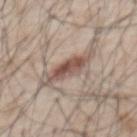| key | value |
|---|---|
| biopsy status | imaged on a skin check; not biopsied |
| patient | male, roughly 65 years of age |
| site | the chest |
| lesion size | ≈5.5 mm |
| acquisition | 15 mm crop, total-body photography |